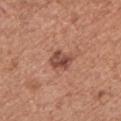This is a white-light tile.
Located on the chest.
A male subject aged approximately 75.
A lesion tile, about 15 mm wide, cut from a 3D total-body photograph.